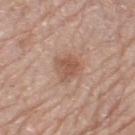The lesion was tiled from a total-body skin photograph and was not biopsied. The lesion is on the left lower leg. The patient is a female about 65 years old. A roughly 15 mm field-of-view crop from a total-body skin photograph. The tile uses white-light illumination.